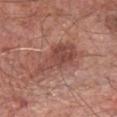Clinical impression: The lesion was tiled from a total-body skin photograph and was not biopsied. Clinical summary: A male subject, aged 63 to 67. A close-up tile cropped from a whole-body skin photograph, about 15 mm across. The tile uses white-light illumination. Measured at roughly 6 mm in maximum diameter. The lesion-visualizer software estimated an area of roughly 13 mm², a shape eccentricity near 0.85, and a symmetry-axis asymmetry near 0.4. And it measured border irregularity of about 5.5 on a 0–10 scale, internal color variation of about 3.5 on a 0–10 scale, and peripheral color asymmetry of about 1.5. And it measured a nevus-likeness score of about 65/100 and lesion-presence confidence of about 100/100. The lesion is located on the chest.